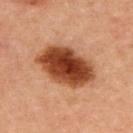{
  "biopsy_status": "not biopsied; imaged during a skin examination",
  "patient": {
    "sex": "female",
    "age_approx": 45
  },
  "site": "upper back",
  "image": {
    "source": "total-body photography crop",
    "field_of_view_mm": 15
  }
}